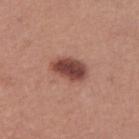Approximately 4 mm at its widest.
A lesion tile, about 15 mm wide, cut from a 3D total-body photograph.
This is a white-light tile.
The lesion is located on the front of the torso.
A female subject in their mid-60s.